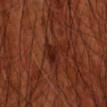Q: Was a biopsy performed?
A: imaged on a skin check; not biopsied
Q: Lesion size?
A: ~2.5 mm (longest diameter)
Q: How was the tile lit?
A: cross-polarized illumination
Q: What kind of image is this?
A: total-body-photography crop, ~15 mm field of view
Q: What are the patient's age and sex?
A: male, about 70 years old
Q: What is the anatomic site?
A: the front of the torso